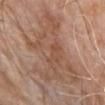follow-up=catalogued during a skin exam; not biopsied | patient=male, aged 78 to 82 | location=the chest | image=~15 mm crop, total-body skin-cancer survey | tile lighting=white-light.